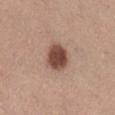No biopsy was performed on this lesion — it was imaged during a full skin examination and was not determined to be concerning. The lesion is located on the right lower leg. A female patient aged around 45. The lesion's longest dimension is about 3.5 mm. A roughly 15 mm field-of-view crop from a total-body skin photograph. An algorithmic analysis of the crop reported a footprint of about 9 mm² and an eccentricity of roughly 0.5. It also reported an average lesion color of about L≈47 a*≈21 b*≈26 (CIELAB) and about 16 CIELAB-L* units darker than the surrounding skin.